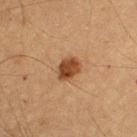Assessment:
Imaged during a routine full-body skin examination; the lesion was not biopsied and no histopathology is available.
Acquisition and patient details:
About 3.5 mm across. The patient is a male about 65 years old. The lesion is located on the left upper arm. A 15 mm close-up extracted from a 3D total-body photography capture. This is a cross-polarized tile. Automated image analysis of the tile measured border irregularity of about 2.5 on a 0–10 scale and internal color variation of about 4 on a 0–10 scale. The software also gave a nevus-likeness score of about 100/100.